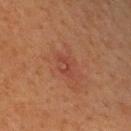| feature | finding |
|---|---|
| notes | catalogued during a skin exam; not biopsied |
| image | total-body-photography crop, ~15 mm field of view |
| illumination | cross-polarized |
| location | the upper back |
| diameter | about 3 mm |
| image-analysis metrics | an area of roughly 3 mm², an eccentricity of roughly 0.85, and a symmetry-axis asymmetry near 0.55; about 6 CIELAB-L* units darker than the surrounding skin and a normalized lesion–skin contrast near 5; a border-irregularity rating of about 6.5/10 and peripheral color asymmetry of about 0; an automated nevus-likeness rating near 0 out of 100 and a lesion-detection confidence of about 100/100 |
| patient | male, about 40 years old |